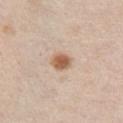From the chest. A 15 mm close-up tile from a total-body photography series done for melanoma screening. The patient is a male roughly 50 years of age.